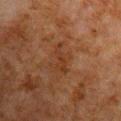This lesion was catalogued during total-body skin photography and was not selected for biopsy.